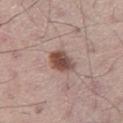Part of a total-body skin-imaging series; this lesion was reviewed on a skin check and was not flagged for biopsy. Longest diameter approximately 3 mm. The patient is a male roughly 60 years of age. The total-body-photography lesion software estimated border irregularity of about 2 on a 0–10 scale, a color-variation rating of about 4.5/10, and a peripheral color-asymmetry measure near 1.5. And it measured a classifier nevus-likeness of about 95/100. Located on the right thigh. A lesion tile, about 15 mm wide, cut from a 3D total-body photograph.